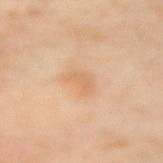Assessment: Captured during whole-body skin photography for melanoma surveillance; the lesion was not biopsied. Clinical summary: Longest diameter approximately 3 mm. A roughly 15 mm field-of-view crop from a total-body skin photograph. The lesion is located on the left upper arm. A female patient aged 53–57. The tile uses cross-polarized illumination.